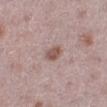The lesion's longest dimension is about 2.5 mm. A female subject in their mid- to late 40s. Automated tile analysis of the lesion measured a lesion area of about 4 mm² and a shape-asymmetry score of about 0.25 (0 = symmetric). And it measured a border-irregularity rating of about 2.5/10. Captured under white-light illumination. Cropped from a whole-body photographic skin survey; the tile spans about 15 mm. Located on the left lower leg.